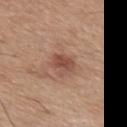| key | value |
|---|---|
| illumination | white-light |
| image | 15 mm crop, total-body photography |
| lesion size | ≈3 mm |
| anatomic site | the mid back |
| subject | male, aged 73 to 77 |